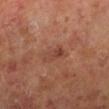Q: Was this lesion biopsied?
A: no biopsy performed (imaged during a skin exam)
Q: Patient demographics?
A: male, roughly 60 years of age
Q: What is the imaging modality?
A: ~15 mm crop, total-body skin-cancer survey
Q: What did automated image analysis measure?
A: an eccentricity of roughly 0.85 and two-axis asymmetry of about 0.25; an average lesion color of about L≈40 a*≈22 b*≈26 (CIELAB), a lesion–skin lightness drop of about 6, and a lesion-to-skin contrast of about 5.5 (normalized; higher = more distinct); internal color variation of about 4.5 on a 0–10 scale and a peripheral color-asymmetry measure near 1.5; an automated nevus-likeness rating near 0 out of 100
Q: Lesion location?
A: the leg